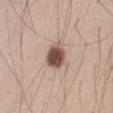Assessment: Captured during whole-body skin photography for melanoma surveillance; the lesion was not biopsied.